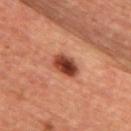<lesion>
  <biopsy_status>not biopsied; imaged during a skin examination</biopsy_status>
  <lesion_size>
    <long_diameter_mm_approx>3.5</long_diameter_mm_approx>
  </lesion_size>
  <patient>
    <sex>female</sex>
    <age_approx>45</age_approx>
  </patient>
  <image>
    <source>total-body photography crop</source>
    <field_of_view_mm>15</field_of_view_mm>
  </image>
  <lighting>cross-polarized</lighting>
  <automated_metrics>
    <area_mm2_approx>7.0</area_mm2_approx>
    <eccentricity>0.8</eccentricity>
    <shape_asymmetry>0.15</shape_asymmetry>
    <vs_skin_darker_L>17.0</vs_skin_darker_L>
    <vs_skin_contrast_norm>12.0</vs_skin_contrast_norm>
    <nevus_likeness_0_100>100</nevus_likeness_0_100>
    <lesion_detection_confidence_0_100>100</lesion_detection_confidence_0_100>
  </automated_metrics>
  <site>upper back</site>
</lesion>